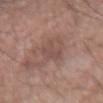| feature | finding |
|---|---|
| follow-up | imaged on a skin check; not biopsied |
| lighting | white-light illumination |
| size | ≈4.5 mm |
| body site | the arm |
| TBP lesion metrics | an area of roughly 4 mm²; a nevus-likeness score of about 0/100 and a detector confidence of about 90 out of 100 that the crop contains a lesion |
| patient | male, in their 50s |
| image | 15 mm crop, total-body photography |